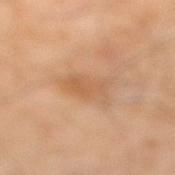Clinical impression: Imaged during a routine full-body skin examination; the lesion was not biopsied and no histopathology is available. Background: The total-body-photography lesion software estimated roughly 7 lightness units darker than nearby skin and a lesion-to-skin contrast of about 5 (normalized; higher = more distinct). The software also gave a classifier nevus-likeness of about 5/100 and lesion-presence confidence of about 100/100. The patient is a male in their mid-60s. Measured at roughly 4 mm in maximum diameter. The lesion is on the right lower leg. A roughly 15 mm field-of-view crop from a total-body skin photograph.